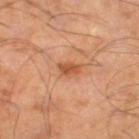The lesion was photographed on a routine skin check and not biopsied; there is no pathology result. A male patient, in their mid-50s. Located on the right thigh. A close-up tile cropped from a whole-body skin photograph, about 15 mm across. The lesion-visualizer software estimated an area of roughly 3.5 mm², an outline eccentricity of about 0.8 (0 = round, 1 = elongated), and a shape-asymmetry score of about 0.25 (0 = symmetric). And it measured a border-irregularity rating of about 2.5/10, a within-lesion color-variation index near 3/10, and radial color variation of about 1. The software also gave a detector confidence of about 100 out of 100 that the crop contains a lesion.